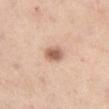Assessment:
Captured during whole-body skin photography for melanoma surveillance; the lesion was not biopsied.
Clinical summary:
The subject is a female approximately 55 years of age. A 15 mm close-up tile from a total-body photography series done for melanoma screening. The lesion's longest dimension is about 3 mm. The total-body-photography lesion software estimated an area of roughly 4.5 mm² and an outline eccentricity of about 0.75 (0 = round, 1 = elongated). It also reported border irregularity of about 1.5 on a 0–10 scale, internal color variation of about 3 on a 0–10 scale, and a peripheral color-asymmetry measure near 1. It also reported a nevus-likeness score of about 95/100 and lesion-presence confidence of about 100/100. The lesion is located on the right thigh. This is a white-light tile.